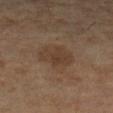Recorded during total-body skin imaging; not selected for excision or biopsy. Located on the left lower leg. A female subject, approximately 70 years of age. This is a cross-polarized tile. Approximately 4.5 mm at its widest. A 15 mm close-up tile from a total-body photography series done for melanoma screening. The total-body-photography lesion software estimated a border-irregularity index near 2.5/10 and a peripheral color-asymmetry measure near 1. The analysis additionally found an automated nevus-likeness rating near 20 out of 100 and a detector confidence of about 100 out of 100 that the crop contains a lesion.